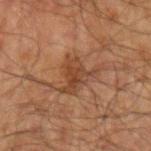This lesion was catalogued during total-body skin photography and was not selected for biopsy. Cropped from a whole-body photographic skin survey; the tile spans about 15 mm. The patient is a male aged around 70. From the arm.